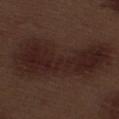This lesion was catalogued during total-body skin photography and was not selected for biopsy.
A male patient aged around 70.
A 15 mm crop from a total-body photograph taken for skin-cancer surveillance.
The lesion is located on the right thigh.
An algorithmic analysis of the crop reported an area of roughly 55 mm² and a symmetry-axis asymmetry near 0.3. The software also gave a lesion color around L≈23 a*≈16 b*≈18 in CIELAB and a lesion–skin lightness drop of about 6. The software also gave a nevus-likeness score of about 5/100 and lesion-presence confidence of about 95/100.
Imaged with white-light lighting.